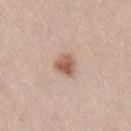Part of a total-body skin-imaging series; this lesion was reviewed on a skin check and was not flagged for biopsy.
The subject is a female about 20 years old.
On the lower back.
A 15 mm crop from a total-body photograph taken for skin-cancer surveillance.
About 3 mm across.